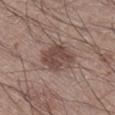This lesion was catalogued during total-body skin photography and was not selected for biopsy.